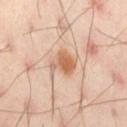image:
  source: total-body photography crop
  field_of_view_mm: 15
automated_metrics:
  cielab_L: 61
  cielab_a: 21
  cielab_b: 35
  vs_skin_darker_L: 11.0
  vs_skin_contrast_norm: 8.5
  border_irregularity_0_10: 3.5
  color_variation_0_10: 3.0
  peripheral_color_asymmetry: 1.0
  nevus_likeness_0_100: 95
  lesion_detection_confidence_0_100: 100
lighting: cross-polarized
site: left thigh
patient:
  sex: male
  age_approx: 45